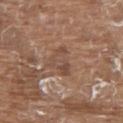A male patient, aged around 80. On the upper back. Captured under white-light illumination. A 15 mm close-up extracted from a 3D total-body photography capture. Approximately 3.5 mm at its widest.